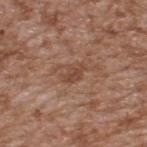The lesion was tiled from a total-body skin photograph and was not biopsied. Captured under white-light illumination. On the upper back. Cropped from a total-body skin-imaging series; the visible field is about 15 mm. The lesion's longest dimension is about 3 mm. The subject is a male aged 63–67.